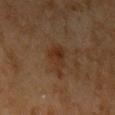automated metrics: a lesion area of about 7.5 mm², an eccentricity of roughly 0.75, and two-axis asymmetry of about 0.4; a lesion color around L≈27 a*≈16 b*≈26 in CIELAB, about 5 CIELAB-L* units darker than the surrounding skin, and a lesion-to-skin contrast of about 6.5 (normalized; higher = more distinct); an automated nevus-likeness rating near 5 out of 100 | illumination: cross-polarized | acquisition: ~15 mm crop, total-body skin-cancer survey | site: the arm | subject: female, aged approximately 70 | lesion size: about 4 mm.